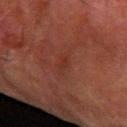Q: Was this lesion biopsied?
A: imaged on a skin check; not biopsied
Q: What lighting was used for the tile?
A: cross-polarized
Q: Automated lesion metrics?
A: a lesion area of about 3 mm², an outline eccentricity of about 0.85 (0 = round, 1 = elongated), and a symmetry-axis asymmetry near 0.25; a classifier nevus-likeness of about 10/100 and lesion-presence confidence of about 100/100
Q: Patient demographics?
A: male, approximately 80 years of age
Q: What is the anatomic site?
A: the left forearm
Q: How large is the lesion?
A: about 2.5 mm
Q: What is the imaging modality?
A: total-body-photography crop, ~15 mm field of view